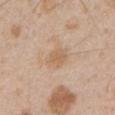Assessment:
The lesion was photographed on a routine skin check and not biopsied; there is no pathology result.
Acquisition and patient details:
The subject is a male aged approximately 50. On the arm. Automated image analysis of the tile measured a symmetry-axis asymmetry near 0.15. The analysis additionally found a classifier nevus-likeness of about 0/100 and a lesion-detection confidence of about 100/100. This is a white-light tile. Measured at roughly 3 mm in maximum diameter. This image is a 15 mm lesion crop taken from a total-body photograph.